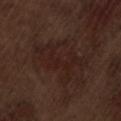notes: no biopsy performed (imaged during a skin exam)
image-analysis metrics: an area of roughly 11 mm², an eccentricity of roughly 0.85, and two-axis asymmetry of about 0.75; about 4 CIELAB-L* units darker than the surrounding skin and a lesion-to-skin contrast of about 5.5 (normalized; higher = more distinct); a border-irregularity rating of about 10/10 and internal color variation of about 2 on a 0–10 scale
lighting: white-light illumination
lesion size: ~6 mm (longest diameter)
body site: the lower back
acquisition: ~15 mm tile from a whole-body skin photo
patient: male, about 70 years old